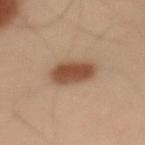Imaged during a routine full-body skin examination; the lesion was not biopsied and no histopathology is available.
Located on the left upper arm.
The subject is a male approximately 55 years of age.
Measured at roughly 4 mm in maximum diameter.
A 15 mm crop from a total-body photograph taken for skin-cancer surveillance.
The tile uses cross-polarized illumination.